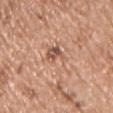Part of a total-body skin-imaging series; this lesion was reviewed on a skin check and was not flagged for biopsy.
Located on the front of the torso.
A close-up tile cropped from a whole-body skin photograph, about 15 mm across.
The subject is a male in their mid-50s.